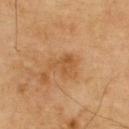follow-up=catalogued during a skin exam; not biopsied
subject=male, aged 68–72
location=the upper back
image source=~15 mm crop, total-body skin-cancer survey